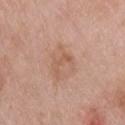No biopsy was performed on this lesion — it was imaged during a full skin examination and was not determined to be concerning. The subject is a male aged around 45. The lesion is located on the upper back. This image is a 15 mm lesion crop taken from a total-body photograph.